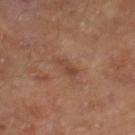biopsy_status: not biopsied; imaged during a skin examination
site: right lower leg
lesion_size:
  long_diameter_mm_approx: 3.0
patient:
  sex: male
  age_approx: 65
automated_metrics:
  area_mm2_approx: 3.5
  cielab_L: 43
  cielab_a: 21
  cielab_b: 28
  vs_skin_darker_L: 7.0
  border_irregularity_0_10: 4.5
  nevus_likeness_0_100: 0
  lesion_detection_confidence_0_100: 100
image:
  source: total-body photography crop
  field_of_view_mm: 15